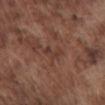subject: male, aged approximately 75 | anatomic site: the front of the torso | imaging modality: ~15 mm tile from a whole-body skin photo | image-analysis metrics: a footprint of about 4.5 mm², an outline eccentricity of about 0.75 (0 = round, 1 = elongated), and two-axis asymmetry of about 0.35; a lesion–skin lightness drop of about 5; lesion-presence confidence of about 90/100 | illumination: white-light.